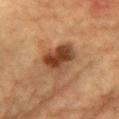Q: Was a biopsy performed?
A: imaged on a skin check; not biopsied
Q: What are the patient's age and sex?
A: male, approximately 85 years of age or older
Q: How was this image acquired?
A: total-body-photography crop, ~15 mm field of view
Q: Where on the body is the lesion?
A: the front of the torso
Q: What did automated image analysis measure?
A: a border-irregularity index near 3.5/10 and a peripheral color-asymmetry measure near 2.5; a nevus-likeness score of about 70/100 and lesion-presence confidence of about 100/100